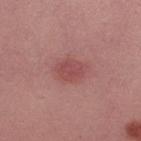Notes:
* notes: imaged on a skin check; not biopsied
* subject: male, aged approximately 40
* tile lighting: white-light illumination
* location: the arm
* image: ~15 mm tile from a whole-body skin photo